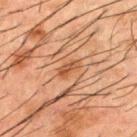Clinical impression:
This lesion was catalogued during total-body skin photography and was not selected for biopsy.
Clinical summary:
A male patient, aged 48 to 52. On the upper back. A 15 mm close-up extracted from a 3D total-body photography capture. Approximately 2.5 mm at its widest. Automated image analysis of the tile measured an area of roughly 3 mm² and a symmetry-axis asymmetry near 0.25. And it measured a lesion color around L≈41 a*≈20 b*≈30 in CIELAB, about 8 CIELAB-L* units darker than the surrounding skin, and a normalized border contrast of about 7. The analysis additionally found an automated nevus-likeness rating near 80 out of 100 and lesion-presence confidence of about 100/100. The tile uses cross-polarized illumination.